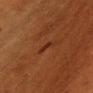Assessment: Imaged during a routine full-body skin examination; the lesion was not biopsied and no histopathology is available. Image and clinical context: A female subject in their mid-50s. On the chest. Imaged with cross-polarized lighting. Cropped from a total-body skin-imaging series; the visible field is about 15 mm. Approximately 2.5 mm at its widest.